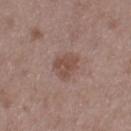Imaged during a routine full-body skin examination; the lesion was not biopsied and no histopathology is available. A 15 mm close-up extracted from a 3D total-body photography capture. From the left thigh. A male subject aged 48–52.